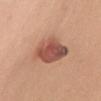Impression:
Recorded during total-body skin imaging; not selected for excision or biopsy.